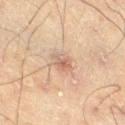notes = catalogued during a skin exam; not biopsied | image = ~15 mm tile from a whole-body skin photo | illumination = cross-polarized illumination | body site = the right thigh | subject = male, aged approximately 70 | size = about 2.5 mm | TBP lesion metrics = an eccentricity of roughly 0.45 and a symmetry-axis asymmetry near 0.2; a border-irregularity index near 2.5/10, a color-variation rating of about 5/10, and a peripheral color-asymmetry measure near 1.5; a nevus-likeness score of about 15/100 and a lesion-detection confidence of about 100/100.